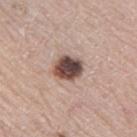workup: catalogued during a skin exam; not biopsied | patient: female, aged approximately 70 | body site: the leg | imaging modality: 15 mm crop, total-body photography.